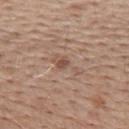biopsy status = no biopsy performed (imaged during a skin exam) | anatomic site = the upper back | imaging modality = 15 mm crop, total-body photography | tile lighting = white-light | patient = male, aged 63 to 67.